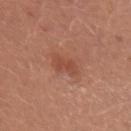notes: imaged on a skin check; not biopsied
imaging modality: ~15 mm crop, total-body skin-cancer survey
illumination: white-light illumination
automated metrics: a footprint of about 4 mm², a shape eccentricity near 0.75, and a shape-asymmetry score of about 0.25 (0 = symmetric); border irregularity of about 3 on a 0–10 scale and a within-lesion color-variation index near 1.5/10; an automated nevus-likeness rating near 5 out of 100 and a lesion-detection confidence of about 100/100
subject: female, in their mid-30s
location: the left upper arm
size: about 2.5 mm